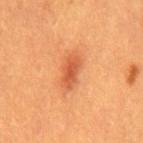Notes:
- follow-up — no biopsy performed (imaged during a skin exam)
- image — total-body-photography crop, ~15 mm field of view
- subject — male, in their 60s
- TBP lesion metrics — a border-irregularity index near 2.5/10, a color-variation rating of about 3/10, and peripheral color asymmetry of about 1
- lighting — cross-polarized illumination
- body site — the mid back